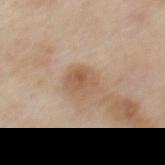This lesion was catalogued during total-body skin photography and was not selected for biopsy.
The tile uses white-light illumination.
Cropped from a total-body skin-imaging series; the visible field is about 15 mm.
A male subject aged around 85 or older.
The lesion is located on the back.